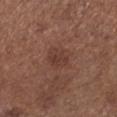Notes:
• acquisition — total-body-photography crop, ~15 mm field of view
• anatomic site — the left lower leg
• patient — female, aged 53–57